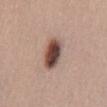Clinical impression:
No biopsy was performed on this lesion — it was imaged during a full skin examination and was not determined to be concerning.
Background:
A close-up tile cropped from a whole-body skin photograph, about 15 mm across. Approximately 5 mm at its widest. Automated tile analysis of the lesion measured a footprint of about 9.5 mm², an eccentricity of roughly 0.85, and two-axis asymmetry of about 0.15. The analysis additionally found border irregularity of about 2 on a 0–10 scale, a within-lesion color-variation index near 6.5/10, and a peripheral color-asymmetry measure near 2. And it measured an automated nevus-likeness rating near 100 out of 100 and lesion-presence confidence of about 100/100. Imaged with white-light lighting. The subject is a male aged around 45. The lesion is on the lower back.